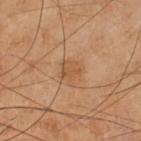Notes:
– notes — no biopsy performed (imaged during a skin exam)
– site — the left lower leg
– size — about 2.5 mm
– illumination — cross-polarized
– patient — male, aged around 65
– image — ~15 mm tile from a whole-body skin photo